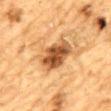acquisition: total-body-photography crop, ~15 mm field of view | patient: male, in their mid- to late 80s or older | location: the mid back | automated metrics: a lesion area of about 12 mm² and an eccentricity of roughly 0.85; a lesion color around L≈47 a*≈22 b*≈37 in CIELAB, about 16 CIELAB-L* units darker than the surrounding skin, and a normalized lesion–skin contrast near 11; border irregularity of about 3 on a 0–10 scale and radial color variation of about 1.5 | illumination: cross-polarized illumination | lesion size: about 5.5 mm.